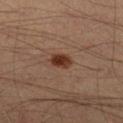No biopsy was performed on this lesion — it was imaged during a full skin examination and was not determined to be concerning.
Located on the right lower leg.
The lesion's longest dimension is about 2.5 mm.
A male patient, aged 38 to 42.
A 15 mm close-up extracted from a 3D total-body photography capture.
Automated image analysis of the tile measured an area of roughly 4 mm² and a shape eccentricity near 0.7. The analysis additionally found a border-irregularity rating of about 2/10 and a color-variation rating of about 3.5/10.
This is a cross-polarized tile.